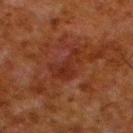notes: total-body-photography surveillance lesion; no biopsy